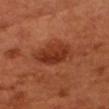biopsy status: imaged on a skin check; not biopsied | imaging modality: ~15 mm crop, total-body skin-cancer survey | anatomic site: the head or neck | lighting: cross-polarized | subject: male, in their 50s.